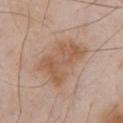{"biopsy_status": "not biopsied; imaged during a skin examination", "lighting": "white-light", "patient": {"sex": "male", "age_approx": 55}, "image": {"source": "total-body photography crop", "field_of_view_mm": 15}, "lesion_size": {"long_diameter_mm_approx": 6.0}, "automated_metrics": {"area_mm2_approx": 20.0, "eccentricity": 0.75, "shape_asymmetry": 0.25, "cielab_L": 57, "cielab_a": 19, "cielab_b": 31, "vs_skin_contrast_norm": 7.0}, "site": "chest"}